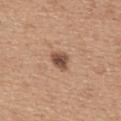follow-up: no biopsy performed (imaged during a skin exam) | patient: male, roughly 65 years of age | diameter: ≈3 mm | body site: the mid back | acquisition: 15 mm crop, total-body photography.